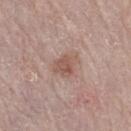Clinical impression: Captured during whole-body skin photography for melanoma surveillance; the lesion was not biopsied. Acquisition and patient details: Located on the right thigh. Measured at roughly 3.5 mm in maximum diameter. A female patient, approximately 65 years of age. Cropped from a whole-body photographic skin survey; the tile spans about 15 mm. This is a white-light tile.